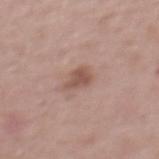Q: Is there a histopathology result?
A: total-body-photography surveillance lesion; no biopsy
Q: What is the anatomic site?
A: the mid back
Q: Patient demographics?
A: male, about 70 years old
Q: What is the imaging modality?
A: ~15 mm tile from a whole-body skin photo